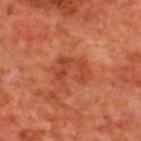Measured at roughly 4 mm in maximum diameter.
A region of skin cropped from a whole-body photographic capture, roughly 15 mm wide.
A male patient, aged approximately 70.
The lesion is located on the back.
This is a cross-polarized tile.
The lesion-visualizer software estimated an area of roughly 9 mm², a shape eccentricity near 0.75, and two-axis asymmetry of about 0.4. The software also gave a lesion color around L≈45 a*≈32 b*≈36 in CIELAB, about 8 CIELAB-L* units darker than the surrounding skin, and a lesion-to-skin contrast of about 6 (normalized; higher = more distinct). And it measured internal color variation of about 3.5 on a 0–10 scale and a peripheral color-asymmetry measure near 1.